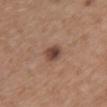biopsy status: no biopsy performed (imaged during a skin exam); subject: female, about 65 years old; lesion diameter: about 2.5 mm; lighting: white-light; image source: ~15 mm crop, total-body skin-cancer survey; location: the chest.